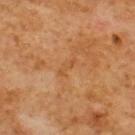The lesion was tiled from a total-body skin photograph and was not biopsied. Captured under cross-polarized illumination. The patient is a male aged approximately 60. On the upper back. A 15 mm close-up tile from a total-body photography series done for melanoma screening. Measured at roughly 2.5 mm in maximum diameter.